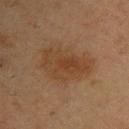| field | value |
|---|---|
| notes | imaged on a skin check; not biopsied |
| patient | male, roughly 55 years of age |
| imaging modality | ~15 mm crop, total-body skin-cancer survey |
| anatomic site | the chest |